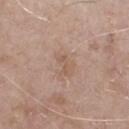Impression:
Captured during whole-body skin photography for melanoma surveillance; the lesion was not biopsied.
Acquisition and patient details:
Longest diameter approximately 2.5 mm. This is a white-light tile. A male patient roughly 65 years of age. Cropped from a whole-body photographic skin survey; the tile spans about 15 mm. From the chest.